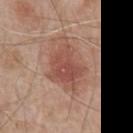Impression: Recorded during total-body skin imaging; not selected for excision or biopsy. Clinical summary: The tile uses white-light illumination. On the chest. A male patient, roughly 80 years of age. The lesion-visualizer software estimated a mean CIELAB color near L≈51 a*≈23 b*≈27, roughly 10 lightness units darker than nearby skin, and a normalized border contrast of about 7. A 15 mm crop from a total-body photograph taken for skin-cancer surveillance.